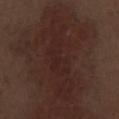follow-up: imaged on a skin check; not biopsied
subject: male, roughly 70 years of age
anatomic site: the left thigh
imaging modality: ~15 mm tile from a whole-body skin photo